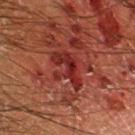Captured during whole-body skin photography for melanoma surveillance; the lesion was not biopsied.
An algorithmic analysis of the crop reported a shape eccentricity near 0.8 and two-axis asymmetry of about 0.35. It also reported an average lesion color of about L≈27 a*≈29 b*≈24 (CIELAB), a lesion–skin lightness drop of about 8, and a lesion-to-skin contrast of about 8 (normalized; higher = more distinct). The software also gave lesion-presence confidence of about 90/100.
Imaged with cross-polarized lighting.
A close-up tile cropped from a whole-body skin photograph, about 15 mm across.
A male patient approximately 65 years of age.
The lesion is on the left lower leg.
The lesion's longest dimension is about 5 mm.